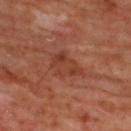Assessment: This lesion was catalogued during total-body skin photography and was not selected for biopsy. Clinical summary: Cropped from a whole-body photographic skin survey; the tile spans about 15 mm. From the back. Measured at roughly 4 mm in maximum diameter. Imaged with cross-polarized lighting. A male patient aged 63–67.